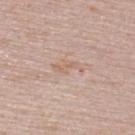The lesion was tiled from a total-body skin photograph and was not biopsied.